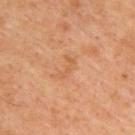follow-up=imaged on a skin check; not biopsied
subject=male, aged 43 to 47
site=the back
lesion size=~3 mm (longest diameter)
illumination=cross-polarized
image source=~15 mm tile from a whole-body skin photo